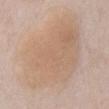This lesion was catalogued during total-body skin photography and was not selected for biopsy. Located on the abdomen. Approximately 12 mm at its widest. Imaged with white-light lighting. The total-body-photography lesion software estimated a mean CIELAB color near L≈64 a*≈16 b*≈30 and about 6 CIELAB-L* units darker than the surrounding skin. And it measured internal color variation of about 3.5 on a 0–10 scale and a peripheral color-asymmetry measure near 1. The analysis additionally found a detector confidence of about 100 out of 100 that the crop contains a lesion. A lesion tile, about 15 mm wide, cut from a 3D total-body photograph. A female subject about 75 years old.